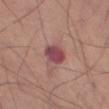| field | value |
|---|---|
| biopsy status | catalogued during a skin exam; not biopsied |
| lesion size | about 3 mm |
| automated metrics | a border-irregularity rating of about 1.5/10, a color-variation rating of about 3.5/10, and peripheral color asymmetry of about 1 |
| anatomic site | the leg |
| acquisition | 15 mm crop, total-body photography |
| patient | male, about 60 years old |
| illumination | white-light |